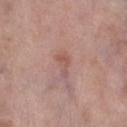<lesion>
<patient>
  <sex>female</sex>
  <age_approx>85+</age_approx>
</patient>
<automated_metrics>
  <area_mm2_approx>3.5</area_mm2_approx>
  <eccentricity>0.8</eccentricity>
  <shape_asymmetry>0.55</shape_asymmetry>
</automated_metrics>
<site>left lower leg</site>
<image>
  <source>total-body photography crop</source>
  <field_of_view_mm>15</field_of_view_mm>
</image>
</lesion>